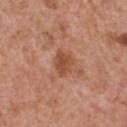Recorded during total-body skin imaging; not selected for excision or biopsy.
A male subject approximately 65 years of age.
A 15 mm crop from a total-body photograph taken for skin-cancer surveillance.
Measured at roughly 3 mm in maximum diameter.
The lesion-visualizer software estimated a footprint of about 5 mm², an outline eccentricity of about 0.6 (0 = round, 1 = elongated), and a shape-asymmetry score of about 0.3 (0 = symmetric). The software also gave a mean CIELAB color near L≈49 a*≈25 b*≈33, about 10 CIELAB-L* units darker than the surrounding skin, and a lesion-to-skin contrast of about 7.5 (normalized; higher = more distinct). The software also gave a within-lesion color-variation index near 2/10 and a peripheral color-asymmetry measure near 0.5. The analysis additionally found an automated nevus-likeness rating near 30 out of 100 and a detector confidence of about 100 out of 100 that the crop contains a lesion.
The lesion is located on the chest.
The tile uses white-light illumination.